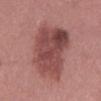Clinical impression: Part of a total-body skin-imaging series; this lesion was reviewed on a skin check and was not flagged for biopsy. Image and clinical context: A 15 mm close-up tile from a total-body photography series done for melanoma screening. An algorithmic analysis of the crop reported a mean CIELAB color near L≈47 a*≈25 b*≈22, a lesion–skin lightness drop of about 12, and a normalized border contrast of about 9. The software also gave border irregularity of about 4 on a 0–10 scale, internal color variation of about 5.5 on a 0–10 scale, and peripheral color asymmetry of about 2. And it measured an automated nevus-likeness rating near 10 out of 100 and a lesion-detection confidence of about 100/100. A female subject aged 38–42. The lesion is located on the leg. Approximately 11.5 mm at its widest.